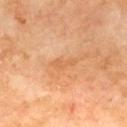Q: Was a biopsy performed?
A: no biopsy performed (imaged during a skin exam)
Q: Patient demographics?
A: male, aged 68 to 72
Q: What kind of image is this?
A: 15 mm crop, total-body photography
Q: What did automated image analysis measure?
A: internal color variation of about 0 on a 0–10 scale and a peripheral color-asymmetry measure near 0; a classifier nevus-likeness of about 0/100 and a lesion-detection confidence of about 100/100
Q: What is the lesion's diameter?
A: ≈3 mm
Q: What is the anatomic site?
A: the mid back